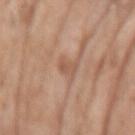Impression: Imaged during a routine full-body skin examination; the lesion was not biopsied and no histopathology is available. Context: The patient is a female aged 83 or older. Located on the right upper arm. A 15 mm close-up tile from a total-body photography series done for melanoma screening.